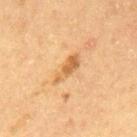Assessment: Recorded during total-body skin imaging; not selected for excision or biopsy. Image and clinical context: From the mid back. A 15 mm close-up tile from a total-body photography series done for melanoma screening. The lesion's longest dimension is about 4.5 mm. The subject is a male aged 58–62. The tile uses cross-polarized illumination.